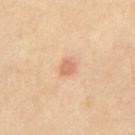{"biopsy_status": "not biopsied; imaged during a skin examination"}